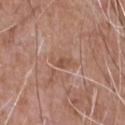Case summary:
• workup: total-body-photography surveillance lesion; no biopsy
• site: the upper back
• patient: male, about 60 years old
• lesion diameter: about 2.5 mm
• image: total-body-photography crop, ~15 mm field of view
• tile lighting: white-light illumination